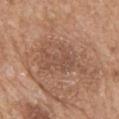No biopsy was performed on this lesion — it was imaged during a full skin examination and was not determined to be concerning. The lesion-visualizer software estimated an area of roughly 15 mm², an eccentricity of roughly 0.85, and a symmetry-axis asymmetry near 0.5. The analysis additionally found a border-irregularity index near 8/10 and a within-lesion color-variation index near 2.5/10. It also reported a nevus-likeness score of about 0/100. This is a white-light tile. The lesion is on the mid back. A roughly 15 mm field-of-view crop from a total-body skin photograph. The subject is a female aged 68–72.